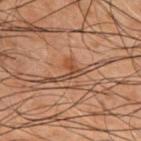Findings:
- biopsy status · total-body-photography surveillance lesion; no biopsy
- body site · the back
- patient · male, aged around 50
- automated lesion analysis · border irregularity of about 5.5 on a 0–10 scale and radial color variation of about 1
- acquisition · ~15 mm tile from a whole-body skin photo
- lesion size · ~2.5 mm (longest diameter)